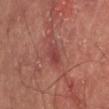Q: Was this lesion biopsied?
A: catalogued during a skin exam; not biopsied